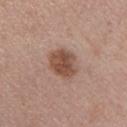<case>
<biopsy_status>not biopsied; imaged during a skin examination</biopsy_status>
<site>chest</site>
<automated_metrics>
  <nevus_likeness_0_100>75</nevus_likeness_0_100>
  <lesion_detection_confidence_0_100>100</lesion_detection_confidence_0_100>
</automated_metrics>
<image>
  <source>total-body photography crop</source>
  <field_of_view_mm>15</field_of_view_mm>
</image>
<patient>
  <sex>male</sex>
  <age_approx>25</age_approx>
</patient>
<lesion_size>
  <long_diameter_mm_approx>4.0</long_diameter_mm_approx>
</lesion_size>
<lighting>white-light</lighting>
</case>